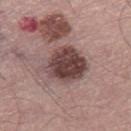<lesion>
<biopsy_status>not biopsied; imaged during a skin examination</biopsy_status>
<patient>
  <sex>male</sex>
  <age_approx>70</age_approx>
</patient>
<lighting>white-light</lighting>
<automated_metrics>
  <area_mm2_approx>18.0</area_mm2_approx>
  <shape_asymmetry>0.15</shape_asymmetry>
  <vs_skin_darker_L>15.0</vs_skin_darker_L>
  <color_variation_0_10>5.5</color_variation_0_10>
  <peripheral_color_asymmetry>2.0</peripheral_color_asymmetry>
</automated_metrics>
<image>
  <source>total-body photography crop</source>
  <field_of_view_mm>15</field_of_view_mm>
</image>
<lesion_size>
  <long_diameter_mm_approx>5.0</long_diameter_mm_approx>
</lesion_size>
<site>right thigh</site>
</lesion>